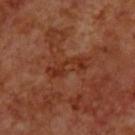Captured during whole-body skin photography for melanoma surveillance; the lesion was not biopsied. Approximately 5 mm at its widest. Located on the upper back. A 15 mm crop from a total-body photograph taken for skin-cancer surveillance. The subject is a male roughly 70 years of age. The tile uses cross-polarized illumination.